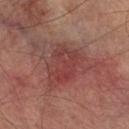| feature | finding |
|---|---|
| notes | no biopsy performed (imaged during a skin exam) |
| image | total-body-photography crop, ~15 mm field of view |
| anatomic site | the leg |
| subject | male, about 75 years old |